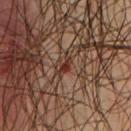Captured during whole-body skin photography for melanoma surveillance; the lesion was not biopsied. The tile uses cross-polarized illumination. A male subject, aged around 65. Automated image analysis of the tile measured a mean CIELAB color near L≈31 a*≈22 b*≈24, roughly 10 lightness units darker than nearby skin, and a lesion-to-skin contrast of about 9.5 (normalized; higher = more distinct). The software also gave an automated nevus-likeness rating near 0 out of 100 and a lesion-detection confidence of about 85/100. A roughly 15 mm field-of-view crop from a total-body skin photograph. The lesion is located on the chest. Measured at roughly 2.5 mm in maximum diameter.